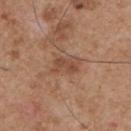Imaged during a routine full-body skin examination; the lesion was not biopsied and no histopathology is available. This image is a 15 mm lesion crop taken from a total-body photograph. Measured at roughly 3 mm in maximum diameter. A male patient, in their mid- to late 50s. Imaged with white-light lighting. Located on the chest.